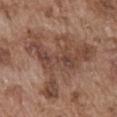Background:
A male subject, aged approximately 75. Cropped from a whole-body photographic skin survey; the tile spans about 15 mm. From the front of the torso. Automated tile analysis of the lesion measured radial color variation of about 1.5. The software also gave a detector confidence of about 95 out of 100 that the crop contains a lesion. Imaged with white-light lighting. Longest diameter approximately 9.5 mm.